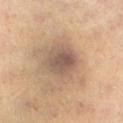Captured during whole-body skin photography for melanoma surveillance; the lesion was not biopsied.
A close-up tile cropped from a whole-body skin photograph, about 15 mm across.
Longest diameter approximately 4 mm.
The lesion is on the left lower leg.
A female patient, aged approximately 40.
Imaged with cross-polarized lighting.